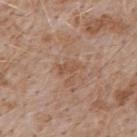<case>
<biopsy_status>not biopsied; imaged during a skin examination</biopsy_status>
<image>
  <source>total-body photography crop</source>
  <field_of_view_mm>15</field_of_view_mm>
</image>
<site>back</site>
<automated_metrics>
  <area_mm2_approx>3.0</area_mm2_approx>
  <cielab_L>52</cielab_L>
  <cielab_a>19</cielab_a>
  <cielab_b>30</cielab_b>
  <vs_skin_darker_L>7.0</vs_skin_darker_L>
  <vs_skin_contrast_norm>5.5</vs_skin_contrast_norm>
  <color_variation_0_10>0.0</color_variation_0_10>
  <peripheral_color_asymmetry>0.0</peripheral_color_asymmetry>
</automated_metrics>
<lesion_size>
  <long_diameter_mm_approx>2.5</long_diameter_mm_approx>
</lesion_size>
<patient>
  <sex>male</sex>
  <age_approx>50</age_approx>
</patient>
<lighting>white-light</lighting>
</case>